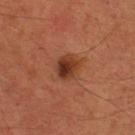{
  "biopsy_status": "not biopsied; imaged during a skin examination",
  "image": {
    "source": "total-body photography crop",
    "field_of_view_mm": 15
  },
  "site": "head or neck",
  "patient": {
    "sex": "male",
    "age_approx": 35
  },
  "lighting": "cross-polarized",
  "lesion_size": {
    "long_diameter_mm_approx": 3.0
  }
}